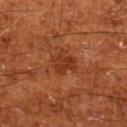Q: Was this lesion biopsied?
A: catalogued during a skin exam; not biopsied
Q: What are the patient's age and sex?
A: male, aged around 65
Q: How was this image acquired?
A: 15 mm crop, total-body photography
Q: How was the tile lit?
A: cross-polarized illumination
Q: What is the anatomic site?
A: the leg